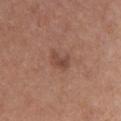| feature | finding |
|---|---|
| biopsy status | no biopsy performed (imaged during a skin exam) |
| lighting | white-light |
| subject | female, approximately 60 years of age |
| acquisition | ~15 mm tile from a whole-body skin photo |
| body site | the back |